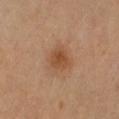The lesion was photographed on a routine skin check and not biopsied; there is no pathology result. The lesion is on the arm. Approximately 3 mm at its widest. Automated image analysis of the tile measured a footprint of about 6 mm². And it measured a mean CIELAB color near L≈47 a*≈21 b*≈34 and a normalized border contrast of about 7. And it measured a border-irregularity rating of about 2.5/10, a color-variation rating of about 2.5/10, and radial color variation of about 1. A roughly 15 mm field-of-view crop from a total-body skin photograph. Imaged with cross-polarized lighting. A female subject, aged 48–52.